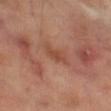Clinical impression: Captured during whole-body skin photography for melanoma surveillance; the lesion was not biopsied. Clinical summary: The lesion is located on the left thigh. A lesion tile, about 15 mm wide, cut from a 3D total-body photograph. A male patient, aged approximately 70.